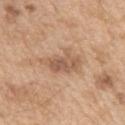Recorded during total-body skin imaging; not selected for excision or biopsy. The subject is a male aged around 70. A close-up tile cropped from a whole-body skin photograph, about 15 mm across. Located on the left upper arm.